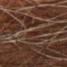Image and clinical context: The subject is a male approximately 80 years of age. Captured under cross-polarized illumination. A 15 mm close-up tile from a total-body photography series done for melanoma screening. The lesion's longest dimension is about 2.5 mm. From the left forearm. Automated tile analysis of the lesion measured a peripheral color-asymmetry measure near 0.5. And it measured a nevus-likeness score of about 0/100 and a detector confidence of about 65 out of 100 that the crop contains a lesion.